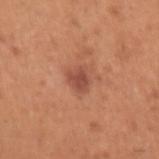This lesion was catalogued during total-body skin photography and was not selected for biopsy.